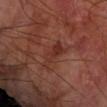<record>
<biopsy_status>not biopsied; imaged during a skin examination</biopsy_status>
<lighting>cross-polarized</lighting>
<automated_metrics>
  <area_mm2_approx>5.5</area_mm2_approx>
  <cielab_L>31</cielab_L>
  <cielab_a>24</cielab_a>
  <cielab_b>26</cielab_b>
  <vs_skin_darker_L>7.0</vs_skin_darker_L>
  <vs_skin_contrast_norm>7.0</vs_skin_contrast_norm>
  <nevus_likeness_0_100>0</nevus_likeness_0_100>
  <lesion_detection_confidence_0_100>95</lesion_detection_confidence_0_100>
</automated_metrics>
<image>
  <source>total-body photography crop</source>
  <field_of_view_mm>15</field_of_view_mm>
</image>
<site>left forearm</site>
<lesion_size>
  <long_diameter_mm_approx>4.5</long_diameter_mm_approx>
</lesion_size>
<patient>
  <sex>male</sex>
  <age_approx>70</age_approx>
</patient>
</record>